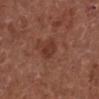Recorded during total-body skin imaging; not selected for excision or biopsy.
The tile uses white-light illumination.
The lesion is located on the left lower leg.
Automated image analysis of the tile measured a border-irregularity index near 1.5/10, internal color variation of about 2 on a 0–10 scale, and radial color variation of about 1.
The patient is a male aged around 75.
The recorded lesion diameter is about 2.5 mm.
A close-up tile cropped from a whole-body skin photograph, about 15 mm across.